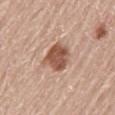| field | value |
|---|---|
| follow-up | total-body-photography surveillance lesion; no biopsy |
| size | about 3.5 mm |
| lighting | white-light illumination |
| patient | male, aged approximately 60 |
| acquisition | ~15 mm tile from a whole-body skin photo |
| site | the mid back |
| TBP lesion metrics | a lesion–skin lightness drop of about 15; a nevus-likeness score of about 75/100 and a detector confidence of about 100 out of 100 that the crop contains a lesion |